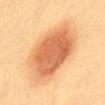This lesion was catalogued during total-body skin photography and was not selected for biopsy. From the abdomen. Cropped from a total-body skin-imaging series; the visible field is about 15 mm. The tile uses cross-polarized illumination. A female subject about 55 years old. An algorithmic analysis of the crop reported border irregularity of about 1.5 on a 0–10 scale, internal color variation of about 4 on a 0–10 scale, and peripheral color asymmetry of about 1. Longest diameter approximately 7.5 mm.